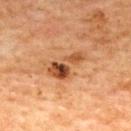Case summary:
– size — ~5 mm (longest diameter)
– anatomic site — the upper back
– patient — female, roughly 60 years of age
– acquisition — ~15 mm crop, total-body skin-cancer survey
– lighting — cross-polarized
– automated lesion analysis — an average lesion color of about L≈40 a*≈22 b*≈32 (CIELAB), a lesion–skin lightness drop of about 12, and a normalized border contrast of about 9.5; a nevus-likeness score of about 55/100 and a lesion-detection confidence of about 100/100